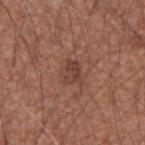lesion_size:
  long_diameter_mm_approx: 2.5
patient:
  sex: male
  age_approx: 60
lighting: white-light
site: arm
image:
  source: total-body photography crop
  field_of_view_mm: 15